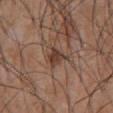Clinical impression: The lesion was tiled from a total-body skin photograph and was not biopsied. Image and clinical context: The lesion-visualizer software estimated an area of roughly 4.5 mm² and an outline eccentricity of about 0.75 (0 = round, 1 = elongated). The analysis additionally found a mean CIELAB color near L≈39 a*≈18 b*≈25, a lesion–skin lightness drop of about 10, and a normalized lesion–skin contrast near 8.5. The lesion's longest dimension is about 3 mm. The patient is a male aged 53–57. A 15 mm crop from a total-body photograph taken for skin-cancer surveillance. The lesion is located on the chest.